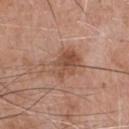workup: catalogued during a skin exam; not biopsied | tile lighting: white-light illumination | subject: male, in their 70s | imaging modality: 15 mm crop, total-body photography | location: the chest | TBP lesion metrics: a footprint of about 11 mm² and an outline eccentricity of about 0.7 (0 = round, 1 = elongated); a lesion color around L≈51 a*≈21 b*≈30 in CIELAB and a lesion-to-skin contrast of about 7 (normalized; higher = more distinct).